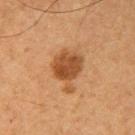The lesion was tiled from a total-body skin photograph and was not biopsied.
A 15 mm close-up extracted from a 3D total-body photography capture.
The lesion is located on the right upper arm.
The subject is a male aged 63 to 67.
This is a cross-polarized tile.